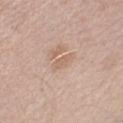follow-up: no biopsy performed (imaged during a skin exam)
image: ~15 mm crop, total-body skin-cancer survey
diameter: about 3 mm
subject: male, roughly 60 years of age
lighting: white-light illumination
anatomic site: the right upper arm